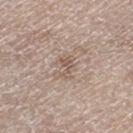Clinical impression: The lesion was tiled from a total-body skin photograph and was not biopsied. Background: Imaged with white-light lighting. A 15 mm close-up extracted from a 3D total-body photography capture. The subject is a male aged 78 to 82. Located on the left thigh. Approximately 2.5 mm at its widest.